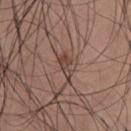biopsy status = imaged on a skin check; not biopsied
patient = male, aged around 35
lighting = white-light illumination
automated metrics = a mean CIELAB color near L≈42 a*≈18 b*≈23 and about 10 CIELAB-L* units darker than the surrounding skin
site = the front of the torso
acquisition = ~15 mm tile from a whole-body skin photo